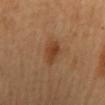Assessment:
The lesion was tiled from a total-body skin photograph and was not biopsied.
Acquisition and patient details:
A female subject in their 60s. The lesion's longest dimension is about 3.5 mm. Captured under cross-polarized illumination. A 15 mm close-up tile from a total-body photography series done for melanoma screening. The lesion-visualizer software estimated an area of roughly 5 mm² and an outline eccentricity of about 0.8 (0 = round, 1 = elongated). The analysis additionally found an average lesion color of about L≈42 a*≈23 b*≈34 (CIELAB). From the mid back.